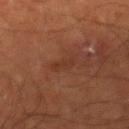Q: Lesion location?
A: the right lower leg
Q: What did automated image analysis measure?
A: a footprint of about 3 mm², an eccentricity of roughly 0.85, and a symmetry-axis asymmetry near 0.35
Q: How was this image acquired?
A: ~15 mm tile from a whole-body skin photo
Q: Patient demographics?
A: male, aged around 65